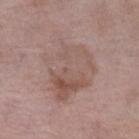biopsy_status: not biopsied; imaged during a skin examination
lesion_size:
  long_diameter_mm_approx: 8.0
patient:
  sex: female
  age_approx: 70
lighting: white-light
image:
  source: total-body photography crop
  field_of_view_mm: 15
site: left lower leg
automated_metrics:
  area_mm2_approx: 29.0
  cielab_L: 54
  cielab_a: 17
  cielab_b: 23
  vs_skin_darker_L: 7.0
  vs_skin_contrast_norm: 5.0
  nevus_likeness_0_100: 0
  lesion_detection_confidence_0_100: 100